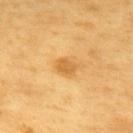Clinical summary:
A lesion tile, about 15 mm wide, cut from a 3D total-body photograph. The subject is a male aged approximately 60. The lesion is on the upper back.